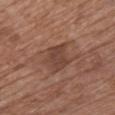Assessment:
Imaged during a routine full-body skin examination; the lesion was not biopsied and no histopathology is available.
Clinical summary:
A female subject about 75 years old. A close-up tile cropped from a whole-body skin photograph, about 15 mm across. The recorded lesion diameter is about 3.5 mm. The tile uses white-light illumination. Located on the front of the torso. The total-body-photography lesion software estimated an average lesion color of about L≈42 a*≈20 b*≈25 (CIELAB), roughly 8 lightness units darker than nearby skin, and a normalized border contrast of about 7. The software also gave border irregularity of about 3.5 on a 0–10 scale, internal color variation of about 2.5 on a 0–10 scale, and peripheral color asymmetry of about 1. It also reported a nevus-likeness score of about 5/100 and lesion-presence confidence of about 100/100.